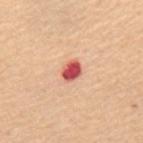The lesion was tiled from a total-body skin photograph and was not biopsied. From the upper back. The lesion's longest dimension is about 2.5 mm. The patient is a female approximately 65 years of age. The tile uses white-light illumination. A 15 mm close-up extracted from a 3D total-body photography capture.